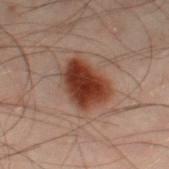No biopsy was performed on this lesion — it was imaged during a full skin examination and was not determined to be concerning.
A male subject, approximately 50 years of age.
Longest diameter approximately 5 mm.
The total-body-photography lesion software estimated a classifier nevus-likeness of about 100/100 and lesion-presence confidence of about 100/100.
The lesion is on the left leg.
This is a cross-polarized tile.
Cropped from a total-body skin-imaging series; the visible field is about 15 mm.